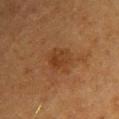| field | value |
|---|---|
| biopsy status | total-body-photography surveillance lesion; no biopsy |
| patient | female, aged around 60 |
| image | total-body-photography crop, ~15 mm field of view |
| automated lesion analysis | a footprint of about 7.5 mm²; a border-irregularity rating of about 2/10 and a color-variation rating of about 3/10; a classifier nevus-likeness of about 0/100 and a lesion-detection confidence of about 100/100 |
| anatomic site | the upper back |
| size | ~3.5 mm (longest diameter) |